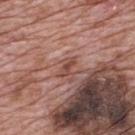{"biopsy_status": "not biopsied; imaged during a skin examination", "site": "mid back", "lighting": "white-light", "lesion_size": {"long_diameter_mm_approx": 2.5}, "image": {"source": "total-body photography crop", "field_of_view_mm": 15}, "automated_metrics": {"nevus_likeness_0_100": 0, "lesion_detection_confidence_0_100": 60}, "patient": {"sex": "male", "age_approx": 70}}